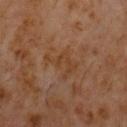Notes:
– workup: imaged on a skin check; not biopsied
– lesion size: ≈4 mm
– subject: male, aged approximately 60
– automated lesion analysis: a nevus-likeness score of about 0/100
– acquisition: ~15 mm tile from a whole-body skin photo
– lighting: cross-polarized illumination
– body site: the chest